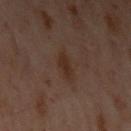Impression: Imaged during a routine full-body skin examination; the lesion was not biopsied and no histopathology is available. Context: Automated tile analysis of the lesion measured an area of roughly 4 mm², an outline eccentricity of about 0.75 (0 = round, 1 = elongated), and a symmetry-axis asymmetry near 0.2. And it measured an average lesion color of about L≈23 a*≈14 b*≈20 (CIELAB), roughly 5 lightness units darker than nearby skin, and a normalized border contrast of about 7. A 15 mm crop from a total-body photograph taken for skin-cancer surveillance. The patient is a female roughly 55 years of age. This is a cross-polarized tile. From the left upper arm.